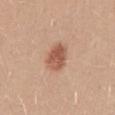| feature | finding |
|---|---|
| biopsy status | catalogued during a skin exam; not biopsied |
| patient | female, roughly 25 years of age |
| lesion diameter | ≈3.5 mm |
| anatomic site | the back |
| lighting | white-light illumination |
| imaging modality | 15 mm crop, total-body photography |